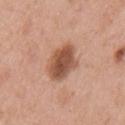Part of a total-body skin-imaging series; this lesion was reviewed on a skin check and was not flagged for biopsy. Automated image analysis of the tile measured a lesion–skin lightness drop of about 15 and a normalized lesion–skin contrast near 10. Cropped from a whole-body photographic skin survey; the tile spans about 15 mm. Imaged with white-light lighting. On the chest. A male patient, aged approximately 55.